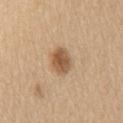Q: Was this lesion biopsied?
A: total-body-photography surveillance lesion; no biopsy
Q: Lesion location?
A: the left upper arm
Q: What lighting was used for the tile?
A: white-light illumination
Q: Patient demographics?
A: female, approximately 60 years of age
Q: How was this image acquired?
A: ~15 mm crop, total-body skin-cancer survey
Q: Lesion size?
A: ~3.5 mm (longest diameter)